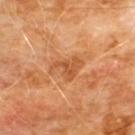biopsy status: total-body-photography surveillance lesion; no biopsy | imaging modality: 15 mm crop, total-body photography | tile lighting: cross-polarized illumination | patient: male, aged 58 to 62 | diameter: ≈4 mm | anatomic site: the chest.